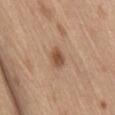notes: catalogued during a skin exam; not biopsied
image source: 15 mm crop, total-body photography
lighting: white-light illumination
diameter: ≈2.5 mm
site: the back
patient: male, aged approximately 70
image-analysis metrics: a lesion area of about 4 mm², an outline eccentricity of about 0.75 (0 = round, 1 = elongated), and two-axis asymmetry of about 0.2; a peripheral color-asymmetry measure near 0.5; a classifier nevus-likeness of about 90/100 and lesion-presence confidence of about 100/100